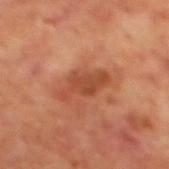<case>
  <biopsy_status>not biopsied; imaged during a skin examination</biopsy_status>
  <image>
    <source>total-body photography crop</source>
    <field_of_view_mm>15</field_of_view_mm>
  </image>
  <patient>
    <sex>male</sex>
    <age_approx>70</age_approx>
  </patient>
  <site>back</site>
  <lesion_size>
    <long_diameter_mm_approx>5.5</long_diameter_mm_approx>
  </lesion_size>
  <lighting>cross-polarized</lighting>
  <automated_metrics>
    <border_irregularity_0_10>4.5</border_irregularity_0_10>
    <color_variation_0_10>2.0</color_variation_0_10>
    <peripheral_color_asymmetry>0.5</peripheral_color_asymmetry>
  </automated_metrics>
</case>